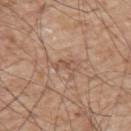Impression:
Part of a total-body skin-imaging series; this lesion was reviewed on a skin check and was not flagged for biopsy.
Acquisition and patient details:
Located on the back. Imaged with white-light lighting. A male subject, aged around 65. A close-up tile cropped from a whole-body skin photograph, about 15 mm across.